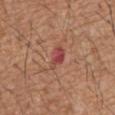Notes:
– follow-up: catalogued during a skin exam; not biopsied
– imaging modality: 15 mm crop, total-body photography
– body site: the abdomen
– patient: male, aged approximately 65
– illumination: white-light
– lesion size: ~3.5 mm (longest diameter)